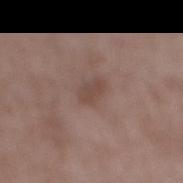The lesion was photographed on a routine skin check and not biopsied; there is no pathology result.
A male patient aged approximately 50.
Measured at roughly 3 mm in maximum diameter.
A close-up tile cropped from a whole-body skin photograph, about 15 mm across.
From the right lower leg.